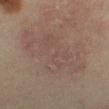Imaged during a routine full-body skin examination; the lesion was not biopsied and no histopathology is available. A roughly 15 mm field-of-view crop from a total-body skin photograph. The patient is a male roughly 65 years of age. The lesion is located on the right thigh.